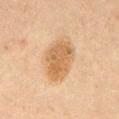Captured during whole-body skin photography for melanoma surveillance; the lesion was not biopsied. The tile uses cross-polarized illumination. On the abdomen. Automated tile analysis of the lesion measured an eccentricity of roughly 0.75. The analysis additionally found a border-irregularity rating of about 1.5/10, internal color variation of about 3 on a 0–10 scale, and a peripheral color-asymmetry measure near 1. And it measured an automated nevus-likeness rating near 65 out of 100. A male patient, aged 58 to 62. Cropped from a total-body skin-imaging series; the visible field is about 15 mm. Approximately 5.5 mm at its widest.